The lesion's longest dimension is about 1.5 mm. The lesion is on the upper back. A female subject, aged 68–72. A lesion tile, about 15 mm wide, cut from a 3D total-body photograph:
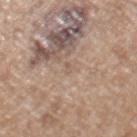{"diagnosis": {"histopathology": "melanoma in situ", "malignancy": "malignant", "taxonomic_path": ["Malignant", "Malignant melanocytic proliferations (Melanoma)", "Melanoma in situ"]}}